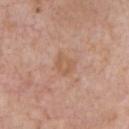This lesion was catalogued during total-body skin photography and was not selected for biopsy. About 2.5 mm across. The lesion is located on the chest. A male subject aged 73–77. Captured under white-light illumination. Cropped from a total-body skin-imaging series; the visible field is about 15 mm.